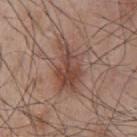workup: no biopsy performed (imaged during a skin exam)
patient: male, aged 63–67
tile lighting: white-light
diameter: ~6.5 mm (longest diameter)
image-analysis metrics: a lesion-detection confidence of about 100/100
site: the chest
acquisition: total-body-photography crop, ~15 mm field of view